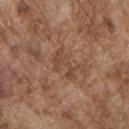Captured during whole-body skin photography for melanoma surveillance; the lesion was not biopsied. A male patient in their mid-70s. Located on the front of the torso. A lesion tile, about 15 mm wide, cut from a 3D total-body photograph.